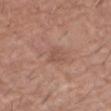Recorded during total-body skin imaging; not selected for excision or biopsy.
From the arm.
Approximately 2.5 mm at its widest.
A male subject, approximately 75 years of age.
This is a white-light tile.
Cropped from a whole-body photographic skin survey; the tile spans about 15 mm.
An algorithmic analysis of the crop reported a shape eccentricity near 0.6 and two-axis asymmetry of about 0.3.